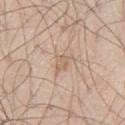Impression: The lesion was photographed on a routine skin check and not biopsied; there is no pathology result. Acquisition and patient details: From the left thigh. Imaged with white-light lighting. A roughly 15 mm field-of-view crop from a total-body skin photograph. A male subject aged 58–62. Longest diameter approximately 3 mm.